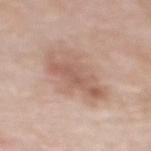Imaged during a routine full-body skin examination; the lesion was not biopsied and no histopathology is available. Longest diameter approximately 6 mm. This is a white-light tile. Automated image analysis of the tile measured a footprint of about 16 mm², an outline eccentricity of about 0.8 (0 = round, 1 = elongated), and a symmetry-axis asymmetry near 0.35. A close-up tile cropped from a whole-body skin photograph, about 15 mm across. On the back. The patient is a female aged 48–52.